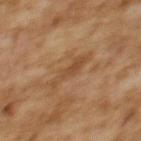Imaged during a routine full-body skin examination; the lesion was not biopsied and no histopathology is available. From the upper back. The patient is a female approximately 60 years of age. A 15 mm crop from a total-body photograph taken for skin-cancer surveillance.